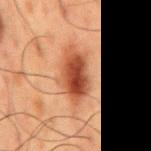follow-up=total-body-photography surveillance lesion; no biopsy